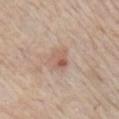Clinical summary: The tile uses white-light illumination. Longest diameter approximately 2.5 mm. A male patient, aged 63 to 67. From the right upper arm. Cropped from a total-body skin-imaging series; the visible field is about 15 mm.